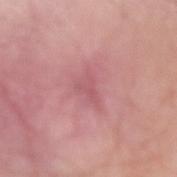Impression:
The lesion was tiled from a total-body skin photograph and was not biopsied.
Background:
A roughly 15 mm field-of-view crop from a total-body skin photograph. The subject is a male aged around 80. The lesion is on the arm. Automated tile analysis of the lesion measured a mean CIELAB color near L≈56 a*≈29 b*≈19, a lesion–skin lightness drop of about 7, and a normalized border contrast of about 4.5. The analysis additionally found border irregularity of about 5.5 on a 0–10 scale, internal color variation of about 1.5 on a 0–10 scale, and peripheral color asymmetry of about 0.5. It also reported a nevus-likeness score of about 0/100 and a detector confidence of about 80 out of 100 that the crop contains a lesion. Measured at roughly 3.5 mm in maximum diameter.